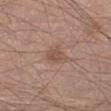Recorded during total-body skin imaging; not selected for excision or biopsy. A 15 mm close-up extracted from a 3D total-body photography capture. Located on the left lower leg. Imaged with white-light lighting. A male patient, in their mid- to late 50s.